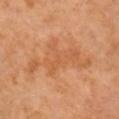Case summary:
– workup · no biopsy performed (imaged during a skin exam)
– imaging modality · ~15 mm crop, total-body skin-cancer survey
– lighting · cross-polarized
– lesion diameter · ~9 mm (longest diameter)
– location · the right arm
– patient · male, in their 60s